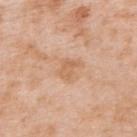The total-body-photography lesion software estimated an outline eccentricity of about 0.7 (0 = round, 1 = elongated) and two-axis asymmetry of about 0.3. This is a white-light tile. On the back. A close-up tile cropped from a whole-body skin photograph, about 15 mm across. A female patient approximately 40 years of age. Measured at roughly 2.5 mm in maximum diameter.